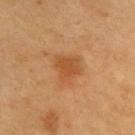notes = imaged on a skin check; not biopsied | imaging modality = total-body-photography crop, ~15 mm field of view | illumination = cross-polarized illumination | size = about 3.5 mm | location = the back | patient = male, aged 58 to 62.